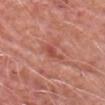<lesion>
  <biopsy_status>not biopsied; imaged during a skin examination</biopsy_status>
  <patient>
    <sex>male</sex>
    <age_approx>80</age_approx>
  </patient>
  <site>chest</site>
  <image>
    <source>total-body photography crop</source>
    <field_of_view_mm>15</field_of_view_mm>
  </image>
  <lighting>white-light</lighting>
  <lesion_size>
    <long_diameter_mm_approx>3.5</long_diameter_mm_approx>
  </lesion_size>
</lesion>